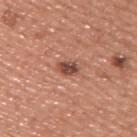No biopsy was performed on this lesion — it was imaged during a full skin examination and was not determined to be concerning.
Located on the upper back.
The subject is a male approximately 45 years of age.
Longest diameter approximately 3 mm.
A 15 mm crop from a total-body photograph taken for skin-cancer surveillance.
Automated tile analysis of the lesion measured a footprint of about 4 mm² and a shape-asymmetry score of about 0.2 (0 = symmetric). And it measured a mean CIELAB color near L≈48 a*≈24 b*≈28, roughly 13 lightness units darker than nearby skin, and a normalized border contrast of about 9.5.